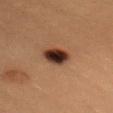A female patient, aged 18 to 22. A 15 mm crop from a total-body photograph taken for skin-cancer surveillance. This is a cross-polarized tile. From the chest.